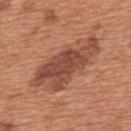Q: Is there a histopathology result?
A: imaged on a skin check; not biopsied
Q: Where on the body is the lesion?
A: the upper back
Q: Illumination type?
A: white-light illumination
Q: Automated lesion metrics?
A: a border-irregularity index near 5.5/10, a color-variation rating of about 5/10, and peripheral color asymmetry of about 1.5; a nevus-likeness score of about 0/100
Q: What kind of image is this?
A: total-body-photography crop, ~15 mm field of view
Q: What is the lesion's diameter?
A: about 9 mm
Q: Who is the patient?
A: male, aged 63 to 67